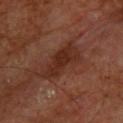No biopsy was performed on this lesion — it was imaged during a full skin examination and was not determined to be concerning.
A male subject, aged 68 to 72.
Cropped from a whole-body photographic skin survey; the tile spans about 15 mm.
On the upper back.